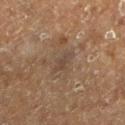follow-up = imaged on a skin check; not biopsied | lesion diameter = ~2.5 mm (longest diameter) | image source = ~15 mm crop, total-body skin-cancer survey | TBP lesion metrics = an automated nevus-likeness rating near 0 out of 100 and lesion-presence confidence of about 90/100 | tile lighting = cross-polarized illumination | location = the left lower leg | patient = male, approximately 75 years of age.